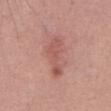workup = no biopsy performed (imaged during a skin exam) | patient = male, aged 53–57 | acquisition = 15 mm crop, total-body photography | diameter = ~5 mm (longest diameter) | location = the abdomen.